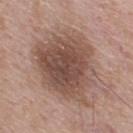notes — total-body-photography surveillance lesion; no biopsy
location — the upper back
lighting — white-light
size — about 8.5 mm
subject — male, aged approximately 50
image-analysis metrics — a lesion area of about 40 mm², an outline eccentricity of about 0.5 (0 = round, 1 = elongated), and a symmetry-axis asymmetry near 0.25; border irregularity of about 3.5 on a 0–10 scale, internal color variation of about 5 on a 0–10 scale, and radial color variation of about 1.5; a nevus-likeness score of about 25/100
image — 15 mm crop, total-body photography